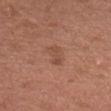Q: Was a biopsy performed?
A: imaged on a skin check; not biopsied
Q: What are the patient's age and sex?
A: male, roughly 25 years of age
Q: Where on the body is the lesion?
A: the front of the torso
Q: What did automated image analysis measure?
A: an area of roughly 5.5 mm² and two-axis asymmetry of about 0.3; border irregularity of about 3.5 on a 0–10 scale, internal color variation of about 2.5 on a 0–10 scale, and a peripheral color-asymmetry measure near 0.5
Q: How was the tile lit?
A: white-light illumination
Q: What is the imaging modality?
A: ~15 mm tile from a whole-body skin photo